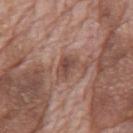Impression:
Recorded during total-body skin imaging; not selected for excision or biopsy.
Context:
A male patient, about 70 years old. A 15 mm close-up extracted from a 3D total-body photography capture. Located on the mid back.